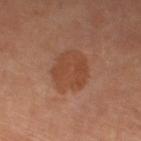A female patient, in their mid-60s. The lesion is located on the leg. A lesion tile, about 15 mm wide, cut from a 3D total-body photograph.